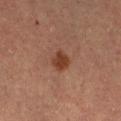Q: Was a biopsy performed?
A: catalogued during a skin exam; not biopsied